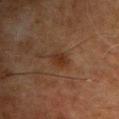Q: Was this lesion biopsied?
A: catalogued during a skin exam; not biopsied
Q: What is the anatomic site?
A: the chest
Q: Patient demographics?
A: male, in their mid- to late 60s
Q: Illumination type?
A: cross-polarized illumination
Q: How was this image acquired?
A: 15 mm crop, total-body photography